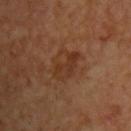Case summary:
• biopsy status: imaged on a skin check; not biopsied
• tile lighting: cross-polarized illumination
• location: the upper back
• lesion diameter: ≈4 mm
• subject: male, aged 68 to 72
• TBP lesion metrics: a lesion area of about 9 mm² and an eccentricity of roughly 0.65; an automated nevus-likeness rating near 0 out of 100 and a detector confidence of about 100 out of 100 that the crop contains a lesion
• acquisition: 15 mm crop, total-body photography